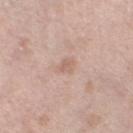No biopsy was performed on this lesion — it was imaged during a full skin examination and was not determined to be concerning.